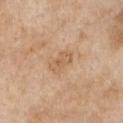This lesion was catalogued during total-body skin photography and was not selected for biopsy. About 3.5 mm across. A close-up tile cropped from a whole-body skin photograph, about 15 mm across. The lesion is located on the chest. Imaged with white-light lighting. The total-body-photography lesion software estimated roughly 7 lightness units darker than nearby skin and a normalized lesion–skin contrast near 5. The analysis additionally found a border-irregularity index near 2.5/10, a within-lesion color-variation index near 2.5/10, and a peripheral color-asymmetry measure near 1. The analysis additionally found lesion-presence confidence of about 100/100. A female subject aged 63 to 67.